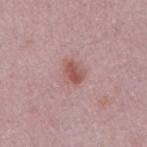Assessment:
Part of a total-body skin-imaging series; this lesion was reviewed on a skin check and was not flagged for biopsy.
Image and clinical context:
Imaged with white-light lighting. A roughly 15 mm field-of-view crop from a total-body skin photograph. Automated tile analysis of the lesion measured a symmetry-axis asymmetry near 0.25. The analysis additionally found a mean CIELAB color near L≈54 a*≈24 b*≈23. The analysis additionally found a within-lesion color-variation index near 3/10. The analysis additionally found an automated nevus-likeness rating near 70 out of 100 and lesion-presence confidence of about 100/100. The patient is a male approximately 25 years of age. From the arm.